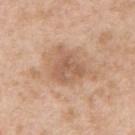Assessment:
Recorded during total-body skin imaging; not selected for excision or biopsy.
Image and clinical context:
Imaged with white-light lighting. A male patient, about 55 years old. The lesion is on the left upper arm. A 15 mm crop from a total-body photograph taken for skin-cancer surveillance.